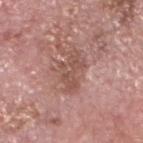The lesion was photographed on a routine skin check and not biopsied; there is no pathology result.
Approximately 4.5 mm at its widest.
A roughly 15 mm field-of-view crop from a total-body skin photograph.
The lesion is located on the head or neck.
The total-body-photography lesion software estimated a mean CIELAB color near L≈52 a*≈21 b*≈25, roughly 9 lightness units darker than nearby skin, and a normalized lesion–skin contrast near 6.
A male subject, approximately 75 years of age.
Captured under white-light illumination.